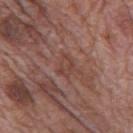Q: Is there a histopathology result?
A: no biopsy performed (imaged during a skin exam)
Q: What kind of image is this?
A: ~15 mm crop, total-body skin-cancer survey
Q: Where on the body is the lesion?
A: the mid back
Q: Who is the patient?
A: male, aged 68 to 72
Q: How was the tile lit?
A: white-light